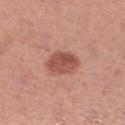Image and clinical context: From the right lower leg. A 15 mm close-up extracted from a 3D total-body photography capture. The subject is a female aged 38–42. Measured at roughly 4 mm in maximum diameter.